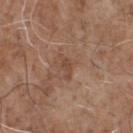Findings:
- biopsy status · total-body-photography surveillance lesion; no biopsy
- diameter · about 3 mm
- patient · male, approximately 70 years of age
- image-analysis metrics · border irregularity of about 4 on a 0–10 scale, a color-variation rating of about 0.5/10, and peripheral color asymmetry of about 0
- body site · the front of the torso
- illumination · white-light illumination
- image · 15 mm crop, total-body photography